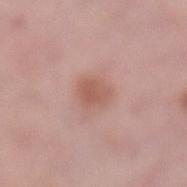workup: catalogued during a skin exam; not biopsied
location: the left lower leg
patient: female, roughly 65 years of age
acquisition: total-body-photography crop, ~15 mm field of view